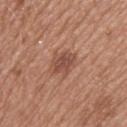Impression: The lesion was photographed on a routine skin check and not biopsied; there is no pathology result. Acquisition and patient details: Longest diameter approximately 3 mm. Cropped from a whole-body photographic skin survey; the tile spans about 15 mm. A male patient, aged around 65. The lesion is located on the upper back.